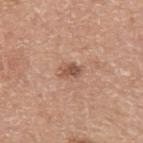workup — catalogued during a skin exam; not biopsied | anatomic site — the arm | lighting — white-light illumination | lesion diameter — ~2.5 mm (longest diameter) | automated lesion analysis — a lesion area of about 3 mm² and two-axis asymmetry of about 0.35; a lesion color around L≈52 a*≈21 b*≈29 in CIELAB, about 11 CIELAB-L* units darker than the surrounding skin, and a normalized lesion–skin contrast near 8; a classifier nevus-likeness of about 30/100 and lesion-presence confidence of about 100/100 | image — 15 mm crop, total-body photography | patient — male, aged around 65.